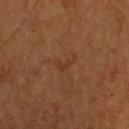Approximately 2.5 mm at its widest. Cropped from a whole-body photographic skin survey; the tile spans about 15 mm. Located on the head or neck. This is a cross-polarized tile. A female patient, in their mid- to late 50s.